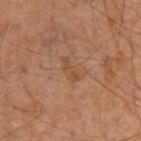biopsy_status: not biopsied; imaged during a skin examination
image:
  source: total-body photography crop
  field_of_view_mm: 15
site: upper back
patient:
  sex: male
  age_approx: 55
automated_metrics:
  cielab_L: 47
  cielab_a: 21
  cielab_b: 32
  vs_skin_darker_L: 6.0
  vs_skin_contrast_norm: 5.5
  border_irregularity_0_10: 5.5
  color_variation_0_10: 0.0
  nevus_likeness_0_100: 0
  lesion_detection_confidence_0_100: 100